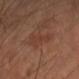<record>
  <biopsy_status>not biopsied; imaged during a skin examination</biopsy_status>
  <lesion_size>
    <long_diameter_mm_approx>5.5</long_diameter_mm_approx>
  </lesion_size>
  <image>
    <source>total-body photography crop</source>
    <field_of_view_mm>15</field_of_view_mm>
  </image>
  <patient>
    <sex>male</sex>
    <age_approx>70</age_approx>
  </patient>
  <site>right forearm</site>
</record>